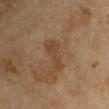This lesion was catalogued during total-body skin photography and was not selected for biopsy.
A male subject, aged 68–72.
The lesion is on the left upper arm.
A roughly 15 mm field-of-view crop from a total-body skin photograph.